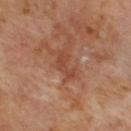Assessment: The lesion was tiled from a total-body skin photograph and was not biopsied. Background: The tile uses cross-polarized illumination. A 15 mm crop from a total-body photograph taken for skin-cancer surveillance. From the back. A male patient, about 70 years old. Measured at roughly 3 mm in maximum diameter.